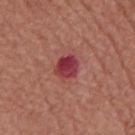Q: Lesion location?
A: the mid back
Q: How was the tile lit?
A: white-light illumination
Q: Who is the patient?
A: male, aged 63–67
Q: What is the lesion's diameter?
A: about 3 mm
Q: What is the imaging modality?
A: total-body-photography crop, ~15 mm field of view